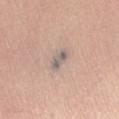Clinical impression:
Part of a total-body skin-imaging series; this lesion was reviewed on a skin check and was not flagged for biopsy.
Background:
A 15 mm close-up tile from a total-body photography series done for melanoma screening. From the lower back. A male subject, aged around 80. The recorded lesion diameter is about 2.5 mm. This is a white-light tile. The lesion-visualizer software estimated a mean CIELAB color near L≈60 a*≈10 b*≈19 and a lesion–skin lightness drop of about 10. And it measured a nevus-likeness score of about 0/100 and a detector confidence of about 80 out of 100 that the crop contains a lesion.